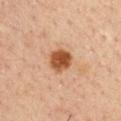Part of a total-body skin-imaging series; this lesion was reviewed on a skin check and was not flagged for biopsy.
Captured under cross-polarized illumination.
The lesion is on the arm.
A 15 mm crop from a total-body photograph taken for skin-cancer surveillance.
The total-body-photography lesion software estimated a footprint of about 7.5 mm², an outline eccentricity of about 0.3 (0 = round, 1 = elongated), and a shape-asymmetry score of about 0.15 (0 = symmetric). The software also gave border irregularity of about 1 on a 0–10 scale, a within-lesion color-variation index near 4/10, and peripheral color asymmetry of about 1.5.
The subject is a male approximately 35 years of age.
Longest diameter approximately 3 mm.